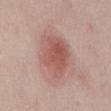  biopsy_status: not biopsied; imaged during a skin examination
  patient:
    sex: female
    age_approx: 40
  site: mid back
  lesion_size:
    long_diameter_mm_approx: 6.0
  automated_metrics:
    area_mm2_approx: 18.0
    eccentricity: 0.75
    shape_asymmetry: 0.2
    cielab_L: 55
    cielab_a: 23
    cielab_b: 24
    vs_skin_darker_L: 11.0
    vs_skin_contrast_norm: 7.5
    border_irregularity_0_10: 2.0
    color_variation_0_10: 4.5
  image:
    source: total-body photography crop
    field_of_view_mm: 15
  lighting: white-light